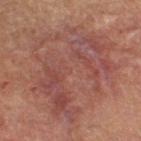A male patient, roughly 65 years of age.
A 15 mm close-up extracted from a 3D total-body photography capture.
The tile uses cross-polarized illumination.
Located on the left thigh.
The recorded lesion diameter is about 11.5 mm.